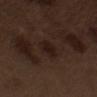The lesion was photographed on a routine skin check and not biopsied; there is no pathology result. A 15 mm close-up tile from a total-body photography series done for melanoma screening. From the arm. The patient is a male aged 68–72.